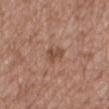Q: Was this lesion biopsied?
A: imaged on a skin check; not biopsied
Q: What is the lesion's diameter?
A: ~2.5 mm (longest diameter)
Q: What lighting was used for the tile?
A: white-light
Q: How was this image acquired?
A: ~15 mm tile from a whole-body skin photo
Q: What are the patient's age and sex?
A: female, about 75 years old
Q: Lesion location?
A: the back